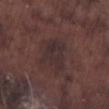workup = imaged on a skin check; not biopsied | body site = the right lower leg | lesion size = ~4.5 mm (longest diameter) | imaging modality = ~15 mm crop, total-body skin-cancer survey | lighting = white-light | automated metrics = a footprint of about 10 mm² and a symmetry-axis asymmetry near 0.4; an average lesion color of about L≈28 a*≈14 b*≈14 (CIELAB), about 5 CIELAB-L* units darker than the surrounding skin, and a normalized lesion–skin contrast near 6.5; a within-lesion color-variation index near 2.5/10 and peripheral color asymmetry of about 1; an automated nevus-likeness rating near 0 out of 100 and a detector confidence of about 90 out of 100 that the crop contains a lesion | subject = male, aged 73 to 77.